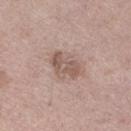The lesion was tiled from a total-body skin photograph and was not biopsied.